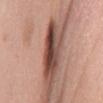follow-up: total-body-photography surveillance lesion; no biopsy | size: about 6.5 mm | illumination: white-light | TBP lesion metrics: a lesion area of about 13 mm², a shape eccentricity near 0.95, and two-axis asymmetry of about 0.25 | acquisition: ~15 mm tile from a whole-body skin photo | patient: female, aged approximately 65 | site: the mid back.